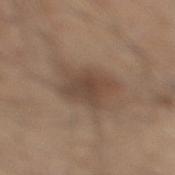The lesion was photographed on a routine skin check and not biopsied; there is no pathology result.
The subject is a male aged approximately 45.
The tile uses white-light illumination.
A 15 mm close-up tile from a total-body photography series done for melanoma screening.
On the right lower leg.